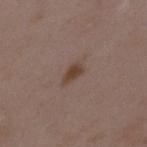Notes:
* follow-up: imaged on a skin check; not biopsied